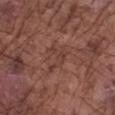About 3.5 mm across. This is a white-light tile. Cropped from a whole-body photographic skin survey; the tile spans about 15 mm. Located on the left forearm. The subject is a male aged approximately 75.